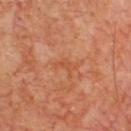The lesion was tiled from a total-body skin photograph and was not biopsied. Measured at roughly 2.5 mm in maximum diameter. Imaged with cross-polarized lighting. A close-up tile cropped from a whole-body skin photograph, about 15 mm across. A male subject, aged 63 to 67. From the chest.